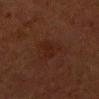Clinical impression:
The lesion was photographed on a routine skin check and not biopsied; there is no pathology result.
Image and clinical context:
Located on the right upper arm. A 15 mm close-up extracted from a 3D total-body photography capture. A female subject roughly 50 years of age.